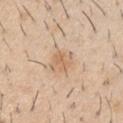This lesion was catalogued during total-body skin photography and was not selected for biopsy. Located on the right upper arm. A male subject aged approximately 60. This image is a 15 mm lesion crop taken from a total-body photograph. The tile uses white-light illumination. Approximately 2.5 mm at its widest.